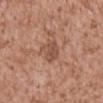Findings:
• follow-up — no biopsy performed (imaged during a skin exam)
• patient — male, about 45 years old
• acquisition — 15 mm crop, total-body photography
• diameter — ≈3.5 mm
• tile lighting — white-light
• body site — the chest